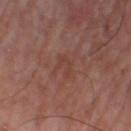– workup: catalogued during a skin exam; not biopsied
– body site: the leg
– image source: total-body-photography crop, ~15 mm field of view